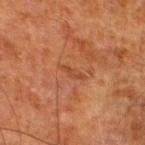follow-up: total-body-photography surveillance lesion; no biopsy
subject: male, in their 80s
image source: 15 mm crop, total-body photography
site: the left lower leg
lesion size: about 3 mm